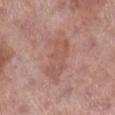Notes:
• notes: no biopsy performed (imaged during a skin exam)
• lesion diameter: about 6.5 mm
• patient: female, aged 48 to 52
• anatomic site: the right lower leg
• image: ~15 mm crop, total-body skin-cancer survey